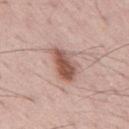Assessment:
Recorded during total-body skin imaging; not selected for excision or biopsy.
Background:
Cropped from a whole-body photographic skin survey; the tile spans about 15 mm. Automated image analysis of the tile measured a nevus-likeness score of about 90/100. A male patient, in their 70s. The lesion is located on the back.